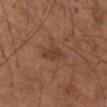• follow-up: imaged on a skin check; not biopsied
• tile lighting: white-light
• lesion diameter: about 4 mm
• subject: male, aged approximately 75
• imaging modality: total-body-photography crop, ~15 mm field of view
• body site: the upper back